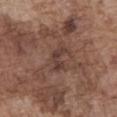  biopsy_status: not biopsied; imaged during a skin examination
  lesion_size:
    long_diameter_mm_approx: 3.5
  lighting: white-light
  patient:
    sex: male
    age_approx: 75
  site: abdomen
  image:
    source: total-body photography crop
    field_of_view_mm: 15